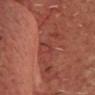The lesion was tiled from a total-body skin photograph and was not biopsied. A close-up tile cropped from a whole-body skin photograph, about 15 mm across. A male patient roughly 60 years of age. The lesion is on the head or neck. The lesion-visualizer software estimated a mean CIELAB color near L≈35 a*≈25 b*≈24 and about 6 CIELAB-L* units darker than the surrounding skin. Measured at roughly 2.5 mm in maximum diameter. Captured under cross-polarized illumination.